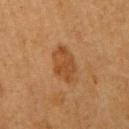Recorded during total-body skin imaging; not selected for excision or biopsy.
A roughly 15 mm field-of-view crop from a total-body skin photograph.
A female subject, about 50 years old.
Located on the right upper arm.
The tile uses cross-polarized illumination.
The recorded lesion diameter is about 4 mm.